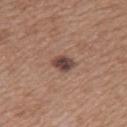workup = no biopsy performed (imaged during a skin exam); site = the left upper arm; subject = female, aged 38 to 42; image source = ~15 mm crop, total-body skin-cancer survey; tile lighting = white-light illumination; diameter = about 2.5 mm.